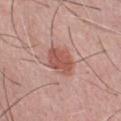<lesion>
<biopsy_status>not biopsied; imaged during a skin examination</biopsy_status>
<lesion_size>
  <long_diameter_mm_approx>4.0</long_diameter_mm_approx>
</lesion_size>
<lighting>white-light</lighting>
<automated_metrics>
  <eccentricity>0.7</eccentricity>
  <shape_asymmetry>0.2</shape_asymmetry>
  <border_irregularity_0_10>2.0</border_irregularity_0_10>
  <peripheral_color_asymmetry>1.0</peripheral_color_asymmetry>
</automated_metrics>
<site>chest</site>
<patient>
  <sex>male</sex>
  <age_approx>50</age_approx>
</patient>
<image>
  <source>total-body photography crop</source>
  <field_of_view_mm>15</field_of_view_mm>
</image>
</lesion>